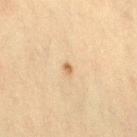{"biopsy_status": "not biopsied; imaged during a skin examination", "image": {"source": "total-body photography crop", "field_of_view_mm": 15}, "site": "right thigh", "lesion_size": {"long_diameter_mm_approx": 1.5}, "patient": {"sex": "female", "age_approx": 40}, "lighting": "cross-polarized"}